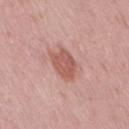notes=total-body-photography surveillance lesion; no biopsy | subject=female, aged 38 to 42 | lesion size=≈4 mm | acquisition=total-body-photography crop, ~15 mm field of view | site=the right thigh.